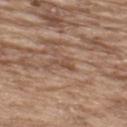Assessment:
The lesion was photographed on a routine skin check and not biopsied; there is no pathology result.
Acquisition and patient details:
Located on the upper back. About 3 mm across. A lesion tile, about 15 mm wide, cut from a 3D total-body photograph. Imaged with white-light lighting. A male subject aged 63–67.